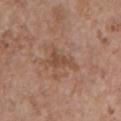Findings:
- follow-up · imaged on a skin check; not biopsied
- tile lighting · white-light
- size · ~4 mm (longest diameter)
- location · the arm
- imaging modality · ~15 mm crop, total-body skin-cancer survey
- subject · female, in their mid-60s
- TBP lesion metrics · an area of roughly 5.5 mm² and two-axis asymmetry of about 0.45; a mean CIELAB color near L≈48 a*≈20 b*≈29, a lesion–skin lightness drop of about 8, and a normalized border contrast of about 6.5; radial color variation of about 0.5; a classifier nevus-likeness of about 0/100 and a detector confidence of about 100 out of 100 that the crop contains a lesion